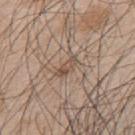lighting: white-light
image:
  source: total-body photography crop
  field_of_view_mm: 15
patient:
  sex: male
  age_approx: 45
site: upper back
automated_metrics:
  cielab_L: 51
  cielab_a: 15
  cielab_b: 26
  vs_skin_contrast_norm: 6.5